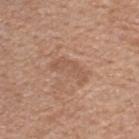The subject is a female aged 48 to 52. Cropped from a total-body skin-imaging series; the visible field is about 15 mm. Captured under white-light illumination. The lesion is on the upper back. Measured at roughly 4.5 mm in maximum diameter.